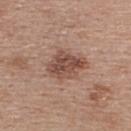Q: Is there a histopathology result?
A: imaged on a skin check; not biopsied
Q: What is the anatomic site?
A: the upper back
Q: What lighting was used for the tile?
A: white-light illumination
Q: What are the patient's age and sex?
A: female, about 40 years old
Q: What kind of image is this?
A: 15 mm crop, total-body photography